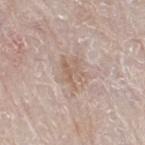Q: Was this lesion biopsied?
A: catalogued during a skin exam; not biopsied
Q: Where on the body is the lesion?
A: the right thigh
Q: Who is the patient?
A: male, aged approximately 80
Q: What lighting was used for the tile?
A: white-light
Q: Lesion size?
A: ~3.5 mm (longest diameter)
Q: What kind of image is this?
A: ~15 mm tile from a whole-body skin photo